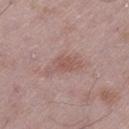* biopsy status — catalogued during a skin exam; not biopsied
* diameter — ≈5 mm
* imaging modality — 15 mm crop, total-body photography
* anatomic site — the left thigh
* subject — male, aged 48–52